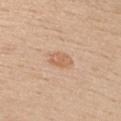{
  "biopsy_status": "not biopsied; imaged during a skin examination",
  "patient": {
    "sex": "male",
    "age_approx": 30
  },
  "automated_metrics": {
    "color_variation_0_10": 2.0,
    "peripheral_color_asymmetry": 0.5,
    "nevus_likeness_0_100": 45
  },
  "image": {
    "source": "total-body photography crop",
    "field_of_view_mm": 15
  },
  "lighting": "white-light",
  "lesion_size": {
    "long_diameter_mm_approx": 3.0
  },
  "site": "chest"
}